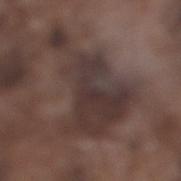A 15 mm close-up extracted from a 3D total-body photography capture. The total-body-photography lesion software estimated a border-irregularity index near 8.5/10, a within-lesion color-variation index near 4.5/10, and radial color variation of about 1. The subject is a male aged 73 to 77. The lesion is on the right lower leg. This is a white-light tile.